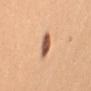No biopsy was performed on this lesion — it was imaged during a full skin examination and was not determined to be concerning.
A region of skin cropped from a whole-body photographic capture, roughly 15 mm wide.
The lesion's longest dimension is about 2.5 mm.
Imaged with white-light lighting.
The patient is a female about 55 years old.
The total-body-photography lesion software estimated internal color variation of about 4.5 on a 0–10 scale and a peripheral color-asymmetry measure near 1.5.